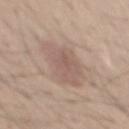biopsy_status: not biopsied; imaged during a skin examination
patient:
  sex: male
  age_approx: 40
lesion_size:
  long_diameter_mm_approx: 7.5
image:
  source: total-body photography crop
  field_of_view_mm: 15
site: mid back